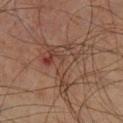Impression: No biopsy was performed on this lesion — it was imaged during a full skin examination and was not determined to be concerning. Clinical summary: Cropped from a total-body skin-imaging series; the visible field is about 15 mm. The lesion is located on the left lower leg. A male subject, aged 58–62.